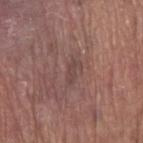<lesion>
  <biopsy_status>not biopsied; imaged during a skin examination</biopsy_status>
  <lesion_size>
    <long_diameter_mm_approx>3.0</long_diameter_mm_approx>
  </lesion_size>
  <lighting>white-light</lighting>
  <site>right upper arm</site>
  <automated_metrics>
    <shape_asymmetry>0.35</shape_asymmetry>
    <nevus_likeness_0_100>0</nevus_likeness_0_100>
  </automated_metrics>
  <image>
    <source>total-body photography crop</source>
    <field_of_view_mm>15</field_of_view_mm>
  </image>
  <patient>
    <sex>male</sex>
    <age_approx>80</age_approx>
  </patient>
</lesion>